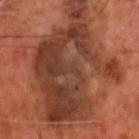Captured during whole-body skin photography for melanoma surveillance; the lesion was not biopsied. A 15 mm close-up tile from a total-body photography series done for melanoma screening. On the head or neck. A male subject aged 68 to 72.